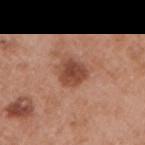follow-up: imaged on a skin check; not biopsied | acquisition: ~15 mm crop, total-body skin-cancer survey | subject: male, aged 53 to 57 | size: about 3.5 mm | image-analysis metrics: a lesion area of about 7.5 mm², a shape eccentricity near 0.5, and a shape-asymmetry score of about 0.2 (0 = symmetric); a mean CIELAB color near L≈46 a*≈24 b*≈30, about 12 CIELAB-L* units darker than the surrounding skin, and a normalized lesion–skin contrast near 8.5; a classifier nevus-likeness of about 75/100 and a lesion-detection confidence of about 100/100 | site: the right upper arm | tile lighting: white-light illumination.